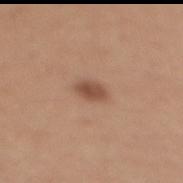Background:
A 15 mm crop from a total-body photograph taken for skin-cancer surveillance. Longest diameter approximately 3 mm. On the chest. Captured under white-light illumination. The subject is a female approximately 35 years of age.